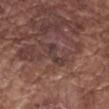Clinical impression:
Imaged during a routine full-body skin examination; the lesion was not biopsied and no histopathology is available.
Acquisition and patient details:
The patient is a male in their mid- to late 70s. Approximately 3.5 mm at its widest. The lesion-visualizer software estimated an average lesion color of about L≈37 a*≈17 b*≈18 (CIELAB), a lesion–skin lightness drop of about 6, and a lesion-to-skin contrast of about 6.5 (normalized; higher = more distinct). It also reported a border-irregularity index near 4.5/10, a within-lesion color-variation index near 1.5/10, and a peripheral color-asymmetry measure near 0.5. And it measured a classifier nevus-likeness of about 0/100 and a lesion-detection confidence of about 80/100. The lesion is located on the right forearm. A 15 mm close-up extracted from a 3D total-body photography capture. Captured under white-light illumination.